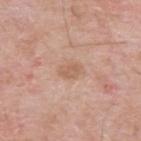workup: catalogued during a skin exam; not biopsied
anatomic site: the upper back
tile lighting: white-light illumination
subject: male, aged approximately 65
imaging modality: total-body-photography crop, ~15 mm field of view
lesion diameter: ≈2.5 mm